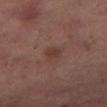Captured during whole-body skin photography for melanoma surveillance; the lesion was not biopsied. Cropped from a total-body skin-imaging series; the visible field is about 15 mm. Captured under cross-polarized illumination. On the right thigh. The patient is a female aged 53 to 57. Longest diameter approximately 2.5 mm.